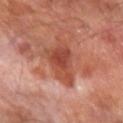<lesion>
<biopsy_status>not biopsied; imaged during a skin examination</biopsy_status>
<image>
  <source>total-body photography crop</source>
  <field_of_view_mm>15</field_of_view_mm>
</image>
<patient>
  <sex>male</sex>
  <age_approx>65</age_approx>
</patient>
<site>left forearm</site>
</lesion>